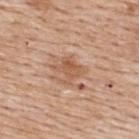Assessment:
Recorded during total-body skin imaging; not selected for excision or biopsy.
Background:
Automated image analysis of the tile measured a footprint of about 5.5 mm² and a shape eccentricity near 0.6. And it measured lesion-presence confidence of about 100/100. Approximately 3.5 mm at its widest. A male patient, aged around 60. The lesion is located on the upper back. This is a white-light tile. Cropped from a total-body skin-imaging series; the visible field is about 15 mm.